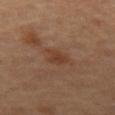The lesion was photographed on a routine skin check and not biopsied; there is no pathology result.
An algorithmic analysis of the crop reported a border-irregularity rating of about 2.5/10, a color-variation rating of about 2/10, and a peripheral color-asymmetry measure near 0.5. The analysis additionally found a nevus-likeness score of about 10/100 and lesion-presence confidence of about 100/100.
About 3 mm across.
Imaged with cross-polarized lighting.
Cropped from a total-body skin-imaging series; the visible field is about 15 mm.
A female subject approximately 60 years of age.
The lesion is on the leg.